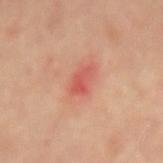This lesion was catalogued during total-body skin photography and was not selected for biopsy. A 15 mm crop from a total-body photograph taken for skin-cancer surveillance. The lesion is located on the lower back. The lesion-visualizer software estimated a footprint of about 4 mm² and an outline eccentricity of about 0.8 (0 = round, 1 = elongated). A male patient roughly 65 years of age. Imaged with cross-polarized lighting. Approximately 3 mm at its widest.